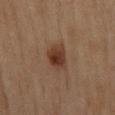acquisition: ~15 mm crop, total-body skin-cancer survey | subject: female, approximately 60 years of age | body site: the leg.